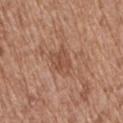Assessment:
Captured during whole-body skin photography for melanoma surveillance; the lesion was not biopsied.
Background:
A region of skin cropped from a whole-body photographic capture, roughly 15 mm wide. Located on the right thigh. A female patient in their mid- to late 70s.